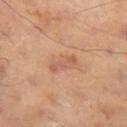Assessment: No biopsy was performed on this lesion — it was imaged during a full skin examination and was not determined to be concerning. Clinical summary: This is a cross-polarized tile. The lesion is located on the leg. Cropped from a total-body skin-imaging series; the visible field is about 15 mm. About 3.5 mm across.